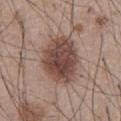Case summary:
– lesion diameter · ≈6 mm
– automated lesion analysis · border irregularity of about 2 on a 0–10 scale, internal color variation of about 5 on a 0–10 scale, and radial color variation of about 1.5
– patient · male, about 55 years old
– body site · the chest
– imaging modality · ~15 mm crop, total-body skin-cancer survey
– illumination · white-light